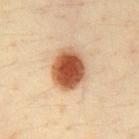{"biopsy_status": "not biopsied; imaged during a skin examination", "image": {"source": "total-body photography crop", "field_of_view_mm": 15}, "patient": {"sex": "male", "age_approx": 35}, "automated_metrics": {"cielab_L": 51, "cielab_a": 23, "cielab_b": 34, "vs_skin_contrast_norm": 12.5}, "lesion_size": {"long_diameter_mm_approx": 5.0}, "lighting": "cross-polarized", "site": "front of the torso"}